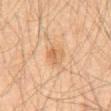The lesion was tiled from a total-body skin photograph and was not biopsied.
Imaged with cross-polarized lighting.
Measured at roughly 2.5 mm in maximum diameter.
A lesion tile, about 15 mm wide, cut from a 3D total-body photograph.
Automated image analysis of the tile measured an eccentricity of roughly 0.45. The software also gave a border-irregularity index near 2.5/10, a color-variation rating of about 4.5/10, and radial color variation of about 1.5. The analysis additionally found a nevus-likeness score of about 40/100 and a lesion-detection confidence of about 100/100.
A male subject aged 58 to 62.
From the front of the torso.